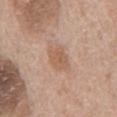Q: Was a biopsy performed?
A: imaged on a skin check; not biopsied
Q: Patient demographics?
A: male, in their 70s
Q: What is the lesion's diameter?
A: about 3.5 mm
Q: What lighting was used for the tile?
A: white-light
Q: What kind of image is this?
A: total-body-photography crop, ~15 mm field of view
Q: Lesion location?
A: the chest